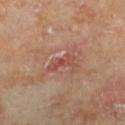The lesion was tiled from a total-body skin photograph and was not biopsied. Cropped from a whole-body photographic skin survey; the tile spans about 15 mm. Imaged with cross-polarized lighting. The subject is a male in their mid-60s. On the left lower leg. Automated tile analysis of the lesion measured an area of roughly 4.5 mm², an outline eccentricity of about 0.9 (0 = round, 1 = elongated), and a symmetry-axis asymmetry near 0.4. And it measured a lesion–skin lightness drop of about 8. It also reported a border-irregularity rating of about 5.5/10 and a color-variation rating of about 0/10. The analysis additionally found a classifier nevus-likeness of about 0/100 and lesion-presence confidence of about 100/100. Measured at roughly 3.5 mm in maximum diameter.